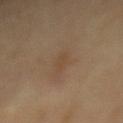biopsy_status: not biopsied; imaged during a skin examination
lighting: cross-polarized
patient:
  sex: male
  age_approx: 60
image:
  source: total-body photography crop
  field_of_view_mm: 15
site: mid back
lesion_size:
  long_diameter_mm_approx: 2.5